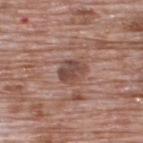This lesion was catalogued during total-body skin photography and was not selected for biopsy. From the back. A male subject, in their 70s. The lesion-visualizer software estimated a shape eccentricity near 0.6 and two-axis asymmetry of about 0.2. The analysis additionally found an average lesion color of about L≈46 a*≈20 b*≈23 (CIELAB) and a lesion–skin lightness drop of about 10. The analysis additionally found a classifier nevus-likeness of about 0/100 and a lesion-detection confidence of about 100/100. This is a white-light tile. Cropped from a total-body skin-imaging series; the visible field is about 15 mm.